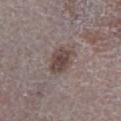{"biopsy_status": "not biopsied; imaged during a skin examination", "lighting": "white-light", "image": {"source": "total-body photography crop", "field_of_view_mm": 15}, "automated_metrics": {"area_mm2_approx": 7.0, "eccentricity": 0.7, "shape_asymmetry": 0.2, "cielab_L": 44, "cielab_a": 14, "cielab_b": 18, "vs_skin_darker_L": 11.0, "vs_skin_contrast_norm": 8.5, "border_irregularity_0_10": 2.5, "color_variation_0_10": 3.5, "peripheral_color_asymmetry": 1.0, "nevus_likeness_0_100": 50, "lesion_detection_confidence_0_100": 100}, "patient": {"sex": "male", "age_approx": 70}, "lesion_size": {"long_diameter_mm_approx": 3.5}, "site": "leg"}